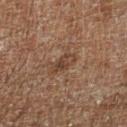Part of a total-body skin-imaging series; this lesion was reviewed on a skin check and was not flagged for biopsy.
The patient is a male aged 58 to 62.
The lesion is located on the leg.
The lesion's longest dimension is about 3.5 mm.
A 15 mm close-up extracted from a 3D total-body photography capture.